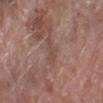follow-up = no biopsy performed (imaged during a skin exam)
site = the arm
image source = 15 mm crop, total-body photography
subject = female, roughly 65 years of age
lighting = white-light illumination
diameter = ~3 mm (longest diameter)